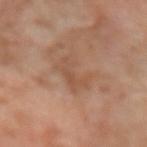Assessment: Captured during whole-body skin photography for melanoma surveillance; the lesion was not biopsied. Clinical summary: A female subject, aged around 50. Cropped from a whole-body photographic skin survey; the tile spans about 15 mm. Measured at roughly 4.5 mm in maximum diameter. Imaged with cross-polarized lighting. The total-body-photography lesion software estimated a lesion area of about 8.5 mm², an eccentricity of roughly 0.85, and a shape-asymmetry score of about 0.45 (0 = symmetric). From the left forearm.